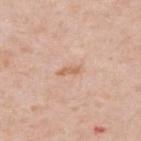workup — no biopsy performed (imaged during a skin exam).